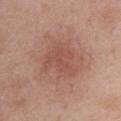Imaged during a routine full-body skin examination; the lesion was not biopsied and no histopathology is available. This image is a 15 mm lesion crop taken from a total-body photograph. Approximately 4.5 mm at its widest. Located on the chest. A female patient approximately 50 years of age.